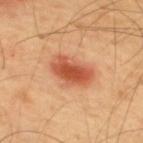Findings:
• notes — imaged on a skin check; not biopsied
• image source — ~15 mm crop, total-body skin-cancer survey
• patient — male, roughly 65 years of age
• automated metrics — an automated nevus-likeness rating near 100 out of 100
• lesion size — ≈5 mm
• lighting — cross-polarized
• body site — the upper back